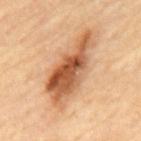{"biopsy_status": "not biopsied; imaged during a skin examination", "lesion_size": {"long_diameter_mm_approx": 9.0}, "patient": {"sex": "male", "age_approx": "85+"}, "site": "mid back", "lighting": "cross-polarized", "image": {"source": "total-body photography crop", "field_of_view_mm": 15}}